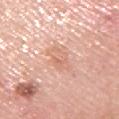Assessment:
Part of a total-body skin-imaging series; this lesion was reviewed on a skin check and was not flagged for biopsy.
Clinical summary:
A region of skin cropped from a whole-body photographic capture, roughly 15 mm wide. A male patient roughly 60 years of age. Located on the right upper arm. Measured at roughly 4 mm in maximum diameter. Captured under white-light illumination.